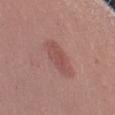The lesion was tiled from a total-body skin photograph and was not biopsied. A female subject in their mid- to late 20s. This image is a 15 mm lesion crop taken from a total-body photograph. The lesion is located on the left upper arm. Imaged with white-light lighting. The lesion-visualizer software estimated a border-irregularity rating of about 3/10, a within-lesion color-variation index near 2/10, and peripheral color asymmetry of about 0.5. And it measured a detector confidence of about 100 out of 100 that the crop contains a lesion.